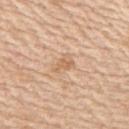Imaged during a routine full-body skin examination; the lesion was not biopsied and no histopathology is available. A roughly 15 mm field-of-view crop from a total-body skin photograph. The lesion's longest dimension is about 3 mm. The lesion is on the upper back. The total-body-photography lesion software estimated an eccentricity of roughly 0.8. The software also gave a color-variation rating of about 2.5/10 and peripheral color asymmetry of about 1. It also reported a lesion-detection confidence of about 100/100. This is a white-light tile. The patient is a male about 60 years old.